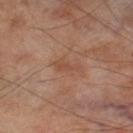biopsy status: catalogued during a skin exam; not biopsied
tile lighting: cross-polarized
automated metrics: a lesion–skin lightness drop of about 6 and a normalized border contrast of about 5; peripheral color asymmetry of about 0; a nevus-likeness score of about 0/100 and a lesion-detection confidence of about 100/100
location: the left thigh
image: ~15 mm tile from a whole-body skin photo
lesion size: about 3 mm
patient: male, approximately 70 years of age